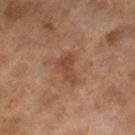{
  "site": "leg",
  "patient": {
    "sex": "female",
    "age_approx": 60
  },
  "automated_metrics": {
    "area_mm2_approx": 6.5,
    "eccentricity": 0.85,
    "cielab_L": 39,
    "cielab_a": 19,
    "cielab_b": 28,
    "color_variation_0_10": 2.5,
    "peripheral_color_asymmetry": 1.0,
    "nevus_likeness_0_100": 0
  },
  "lighting": "cross-polarized",
  "image": {
    "source": "total-body photography crop",
    "field_of_view_mm": 15
  }
}